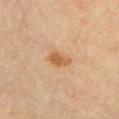{
  "biopsy_status": "not biopsied; imaged during a skin examination",
  "lesion_size": {
    "long_diameter_mm_approx": 3.0
  },
  "lighting": "cross-polarized",
  "image": {
    "source": "total-body photography crop",
    "field_of_view_mm": 15
  },
  "patient": {
    "sex": "male",
    "age_approx": 65
  },
  "site": "upper back"
}